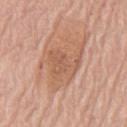subject: male, approximately 80 years of age; lesion size: ≈7 mm; imaging modality: 15 mm crop, total-body photography; tile lighting: white-light illumination; site: the chest.